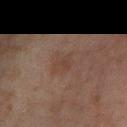patient:
  sex: male
  age_approx: 45
image:
  source: total-body photography crop
  field_of_view_mm: 15
lesion_size:
  long_diameter_mm_approx: 2.5
site: left forearm
lighting: cross-polarized